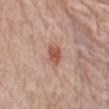<case>
<biopsy_status>not biopsied; imaged during a skin examination</biopsy_status>
<image>
  <source>total-body photography crop</source>
  <field_of_view_mm>15</field_of_view_mm>
</image>
<lighting>white-light</lighting>
<site>left forearm</site>
<patient>
  <sex>male</sex>
  <age_approx>75</age_approx>
</patient>
<lesion_size>
  <long_diameter_mm_approx>2.5</long_diameter_mm_approx>
</lesion_size>
</case>